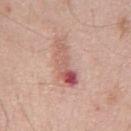Impression: Recorded during total-body skin imaging; not selected for excision or biopsy. Clinical summary: Captured under white-light illumination. The lesion is located on the chest. The patient is a male aged 53 to 57. A 15 mm crop from a total-body photograph taken for skin-cancer surveillance. Approximately 6 mm at its widest.